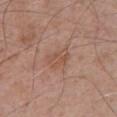Clinical summary: A lesion tile, about 15 mm wide, cut from a 3D total-body photograph. A male patient, about 70 years old. The lesion is on the chest.